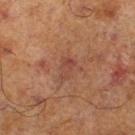Imaged during a routine full-body skin examination; the lesion was not biopsied and no histopathology is available. The recorded lesion diameter is about 2.5 mm. The tile uses cross-polarized illumination. A 15 mm crop from a total-body photograph taken for skin-cancer surveillance. The lesion is on the right lower leg. The total-body-photography lesion software estimated a footprint of about 3 mm², an outline eccentricity of about 0.85 (0 = round, 1 = elongated), and two-axis asymmetry of about 0.4. It also reported a lesion–skin lightness drop of about 5. The analysis additionally found a border-irregularity rating of about 4.5/10, a color-variation rating of about 1/10, and a peripheral color-asymmetry measure near 0.5. And it measured a nevus-likeness score of about 0/100 and lesion-presence confidence of about 100/100. The subject is a male aged 68–72.